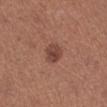Imaged during a routine full-body skin examination; the lesion was not biopsied and no histopathology is available.
Automated tile analysis of the lesion measured a footprint of about 5.5 mm² and a symmetry-axis asymmetry near 0.2.
A female subject roughly 35 years of age.
A lesion tile, about 15 mm wide, cut from a 3D total-body photograph.
Located on the left lower leg.